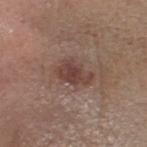Findings:
- biopsy status · no biopsy performed (imaged during a skin exam)
- location · the head or neck
- size · about 4 mm
- image · total-body-photography crop, ~15 mm field of view
- patient · female, approximately 65 years of age